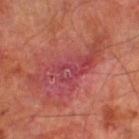No biopsy was performed on this lesion — it was imaged during a full skin examination and was not determined to be concerning. The tile uses cross-polarized illumination. The patient is a male about 70 years old. A 15 mm close-up extracted from a 3D total-body photography capture. Located on the right thigh.